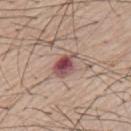Imaged during a routine full-body skin examination; the lesion was not biopsied and no histopathology is available.
The total-body-photography lesion software estimated an average lesion color of about L≈50 a*≈23 b*≈20 (CIELAB), a lesion–skin lightness drop of about 14, and a normalized border contrast of about 10. And it measured a nevus-likeness score of about 0/100.
About 4 mm across.
A lesion tile, about 15 mm wide, cut from a 3D total-body photograph.
The tile uses white-light illumination.
A male subject, roughly 55 years of age.